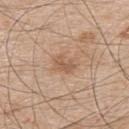<record>
  <biopsy_status>not biopsied; imaged during a skin examination</biopsy_status>
  <lighting>white-light</lighting>
  <automated_metrics>
    <shape_asymmetry>0.3</shape_asymmetry>
    <border_irregularity_0_10>3.0</border_irregularity_0_10>
    <color_variation_0_10>1.0</color_variation_0_10>
    <peripheral_color_asymmetry>0.5</peripheral_color_asymmetry>
  </automated_metrics>
  <patient>
    <sex>male</sex>
    <age_approx>50</age_approx>
  </patient>
  <image>
    <source>total-body photography crop</source>
    <field_of_view_mm>15</field_of_view_mm>
  </image>
  <site>upper back</site>
</record>